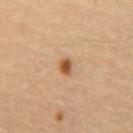This lesion was catalogued during total-body skin photography and was not selected for biopsy. A region of skin cropped from a whole-body photographic capture, roughly 15 mm wide. The lesion is located on the abdomen. The tile uses cross-polarized illumination. A male patient, roughly 55 years of age. Longest diameter approximately 2 mm.